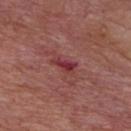Q: Was a biopsy performed?
A: imaged on a skin check; not biopsied
Q: What is the imaging modality?
A: total-body-photography crop, ~15 mm field of view
Q: How large is the lesion?
A: about 3 mm
Q: Patient demographics?
A: male, aged 73 to 77
Q: Where on the body is the lesion?
A: the chest
Q: What lighting was used for the tile?
A: white-light